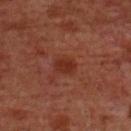Q: Is there a histopathology result?
A: no biopsy performed (imaged during a skin exam)
Q: What is the imaging modality?
A: 15 mm crop, total-body photography
Q: What lighting was used for the tile?
A: cross-polarized
Q: Lesion location?
A: the chest
Q: Patient demographics?
A: male, aged approximately 60
Q: Lesion size?
A: ≈2.5 mm
Q: What did automated image analysis measure?
A: a footprint of about 4.5 mm², a shape eccentricity near 0.65, and a symmetry-axis asymmetry near 0.15; border irregularity of about 1.5 on a 0–10 scale, a within-lesion color-variation index near 2/10, and peripheral color asymmetry of about 0.5; a nevus-likeness score of about 45/100 and a detector confidence of about 100 out of 100 that the crop contains a lesion